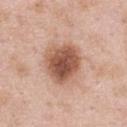Clinical impression: Imaged during a routine full-body skin examination; the lesion was not biopsied and no histopathology is available. Acquisition and patient details: The total-body-photography lesion software estimated a detector confidence of about 100 out of 100 that the crop contains a lesion. Approximately 5 mm at its widest. The lesion is on the left upper arm. A 15 mm crop from a total-body photograph taken for skin-cancer surveillance. A male patient approximately 55 years of age. Imaged with white-light lighting.